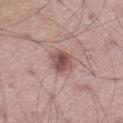Captured during whole-body skin photography for melanoma surveillance; the lesion was not biopsied. Longest diameter approximately 3 mm. Captured under white-light illumination. The lesion-visualizer software estimated a lesion area of about 6 mm², an outline eccentricity of about 0.5 (0 = round, 1 = elongated), and a shape-asymmetry score of about 0.2 (0 = symmetric). It also reported an automated nevus-likeness rating near 75 out of 100 and lesion-presence confidence of about 100/100. A male patient aged 53–57. The lesion is located on the left thigh. A 15 mm close-up extracted from a 3D total-body photography capture.